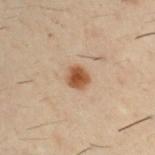Q: Was a biopsy performed?
A: no biopsy performed (imaged during a skin exam)
Q: What is the anatomic site?
A: the right upper arm
Q: Patient demographics?
A: male, aged 28–32
Q: How was the tile lit?
A: cross-polarized illumination
Q: How was this image acquired?
A: 15 mm crop, total-body photography